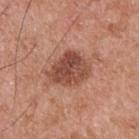Impression:
The lesion was photographed on a routine skin check and not biopsied; there is no pathology result.
Context:
A 15 mm close-up extracted from a 3D total-body photography capture. On the upper back. A male subject, aged approximately 55. Longest diameter approximately 4.5 mm.